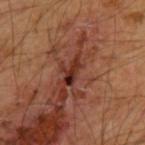biopsy status = no biopsy performed (imaged during a skin exam); body site = the upper back; image = ~15 mm crop, total-body skin-cancer survey; tile lighting = cross-polarized illumination; patient = male, approximately 55 years of age; diameter = ≈3.5 mm.